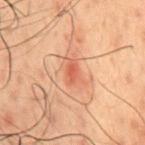Clinical impression:
Recorded during total-body skin imaging; not selected for excision or biopsy.
Image and clinical context:
The lesion is located on the chest. The lesion's longest dimension is about 2.5 mm. A male patient aged 48 to 52. A 15 mm close-up extracted from a 3D total-body photography capture.